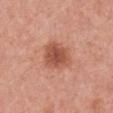Imaged during a routine full-body skin examination; the lesion was not biopsied and no histopathology is available. A female subject, aged approximately 35. A roughly 15 mm field-of-view crop from a total-body skin photograph. From the chest. Automated image analysis of the tile measured an outline eccentricity of about 0.5 (0 = round, 1 = elongated) and two-axis asymmetry of about 0.2. And it measured a nevus-likeness score of about 75/100 and lesion-presence confidence of about 100/100. Captured under white-light illumination.